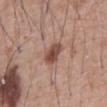Case summary:
- workup: catalogued during a skin exam; not biopsied
- imaging modality: ~15 mm tile from a whole-body skin photo
- body site: the front of the torso
- patient: male, aged 58 to 62